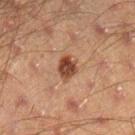| key | value |
|---|---|
| biopsy status | catalogued during a skin exam; not biopsied |
| lesion size | ≈2.5 mm |
| location | the right lower leg |
| patient | male, aged approximately 45 |
| automated metrics | a border-irregularity rating of about 2/10 and radial color variation of about 1.5 |
| illumination | cross-polarized |
| acquisition | ~15 mm crop, total-body skin-cancer survey |